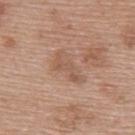Case summary:
* biopsy status: imaged on a skin check; not biopsied
* location: the upper back
* illumination: white-light illumination
* subject: female, aged 58 to 62
* imaging modality: ~15 mm tile from a whole-body skin photo
* TBP lesion metrics: an eccentricity of roughly 0.9; about 7 CIELAB-L* units darker than the surrounding skin; a border-irregularity index near 4.5/10 and a within-lesion color-variation index near 3/10